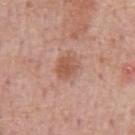This lesion was catalogued during total-body skin photography and was not selected for biopsy. An algorithmic analysis of the crop reported a lesion area of about 5.5 mm², an outline eccentricity of about 0.55 (0 = round, 1 = elongated), and a shape-asymmetry score of about 0.15 (0 = symmetric). The software also gave about 9 CIELAB-L* units darker than the surrounding skin and a lesion-to-skin contrast of about 7 (normalized; higher = more distinct). The analysis additionally found a border-irregularity index near 1.5/10, a color-variation rating of about 2.5/10, and a peripheral color-asymmetry measure near 1. A male subject, about 60 years old. Imaged with white-light lighting. The lesion is located on the front of the torso. About 3 mm across. A 15 mm close-up tile from a total-body photography series done for melanoma screening.